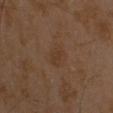Imaged during a routine full-body skin examination; the lesion was not biopsied and no histopathology is available. The lesion's longest dimension is about 2.5 mm. Automated tile analysis of the lesion measured a lesion color around L≈32 a*≈15 b*≈26 in CIELAB, a lesion–skin lightness drop of about 4, and a lesion-to-skin contrast of about 5.5 (normalized; higher = more distinct). The software also gave a border-irregularity rating of about 4/10 and a within-lesion color-variation index near 0.5/10. The analysis additionally found an automated nevus-likeness rating near 0 out of 100 and a detector confidence of about 100 out of 100 that the crop contains a lesion. Captured under cross-polarized illumination. On the head or neck. A 15 mm crop from a total-body photograph taken for skin-cancer surveillance. A male subject aged approximately 60.